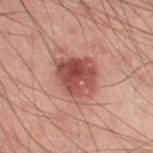notes=total-body-photography surveillance lesion; no biopsy
subject=male, aged 38–42
illumination=cross-polarized
anatomic site=the left thigh
image-analysis metrics=an area of roughly 15 mm², an eccentricity of roughly 0.55, and a symmetry-axis asymmetry near 0.2; an average lesion color of about L≈39 a*≈22 b*≈22 (CIELAB) and a lesion–skin lightness drop of about 11; border irregularity of about 2.5 on a 0–10 scale, a within-lesion color-variation index near 6.5/10, and radial color variation of about 2.5; a classifier nevus-likeness of about 85/100 and a lesion-detection confidence of about 100/100
image=15 mm crop, total-body photography